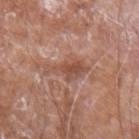Part of a total-body skin-imaging series; this lesion was reviewed on a skin check and was not flagged for biopsy. Located on the left forearm. A 15 mm crop from a total-body photograph taken for skin-cancer surveillance. A male subject aged around 60.